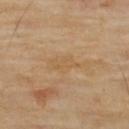Clinical impression: Part of a total-body skin-imaging series; this lesion was reviewed on a skin check and was not flagged for biopsy. Image and clinical context: A 15 mm close-up extracted from a 3D total-body photography capture. The subject is a male approximately 65 years of age. The lesion is on the upper back. Approximately 4 mm at its widest. The tile uses cross-polarized illumination.